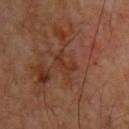location: the upper back | automated lesion analysis: a footprint of about 4 mm², an eccentricity of roughly 0.85, and a symmetry-axis asymmetry near 0.55; roughly 5 lightness units darker than nearby skin; a classifier nevus-likeness of about 0/100 and a detector confidence of about 95 out of 100 that the crop contains a lesion | lighting: cross-polarized | image source: ~15 mm tile from a whole-body skin photo | lesion diameter: ~3 mm (longest diameter) | patient: male, roughly 60 years of age.